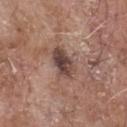biopsy_status: not biopsied; imaged during a skin examination
lesion_size:
  long_diameter_mm_approx: 4.0
automated_metrics:
  area_mm2_approx: 8.0
  eccentricity: 0.8
  shape_asymmetry: 0.2
  border_irregularity_0_10: 2.5
  color_variation_0_10: 6.5
  peripheral_color_asymmetry: 2.0
  nevus_likeness_0_100: 5
  lesion_detection_confidence_0_100: 100
site: chest
lighting: white-light
image:
  source: total-body photography crop
  field_of_view_mm: 15
patient:
  sex: male
  age_approx: 70